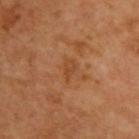- notes: catalogued during a skin exam; not biopsied
- body site: the upper back
- image source: 15 mm crop, total-body photography
- lighting: cross-polarized
- subject: male, aged 63–67